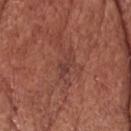The tile uses white-light illumination. From the head or neck. The total-body-photography lesion software estimated border irregularity of about 4.5 on a 0–10 scale and a color-variation rating of about 0/10. The analysis additionally found a nevus-likeness score of about 0/100. A lesion tile, about 15 mm wide, cut from a 3D total-body photograph. The patient is a male aged 63–67.